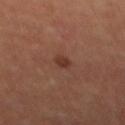Clinical impression: No biopsy was performed on this lesion — it was imaged during a full skin examination and was not determined to be concerning. Acquisition and patient details: This image is a 15 mm lesion crop taken from a total-body photograph. Longest diameter approximately 2 mm. The lesion is on the abdomen. Automated image analysis of the tile measured a footprint of about 2.5 mm² and a shape-asymmetry score of about 0.15 (0 = symmetric). And it measured a classifier nevus-likeness of about 95/100 and a detector confidence of about 100 out of 100 that the crop contains a lesion. A male patient aged 63 to 67.